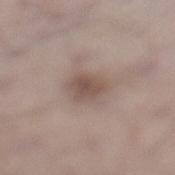No biopsy was performed on this lesion — it was imaged during a full skin examination and was not determined to be concerning.